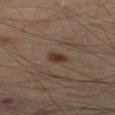Q: Was a biopsy performed?
A: imaged on a skin check; not biopsied
Q: Lesion location?
A: the left thigh
Q: What kind of image is this?
A: ~15 mm crop, total-body skin-cancer survey
Q: What did automated image analysis measure?
A: a mean CIELAB color near L≈35 a*≈18 b*≈26 and roughly 10 lightness units darker than nearby skin; a border-irregularity index near 2/10 and radial color variation of about 0.5
Q: Who is the patient?
A: male, aged around 55
Q: What is the lesion's diameter?
A: about 2.5 mm
Q: Illumination type?
A: cross-polarized